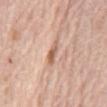Part of a total-body skin-imaging series; this lesion was reviewed on a skin check and was not flagged for biopsy. The lesion is located on the abdomen. The subject is a female aged 63–67. Imaged with white-light lighting. A 15 mm close-up tile from a total-body photography series done for melanoma screening. Measured at roughly 3.5 mm in maximum diameter. Automated tile analysis of the lesion measured a lesion area of about 4 mm², an outline eccentricity of about 0.95 (0 = round, 1 = elongated), and two-axis asymmetry of about 0.4. The software also gave a mean CIELAB color near L≈62 a*≈21 b*≈30. The analysis additionally found a border-irregularity rating of about 4/10 and a color-variation rating of about 4/10.